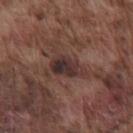{"biopsy_status": "not biopsied; imaged during a skin examination", "patient": {"sex": "male", "age_approx": 75}, "image": {"source": "total-body photography crop", "field_of_view_mm": 15}, "site": "front of the torso"}